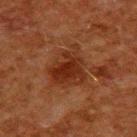Context:
A male subject in their 60s. Longest diameter approximately 5 mm. A 15 mm crop from a total-body photograph taken for skin-cancer surveillance. The tile uses cross-polarized illumination. From the upper back.